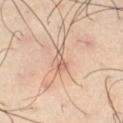follow-up = imaged on a skin check; not biopsied | body site = the right thigh | subject = male, aged 38 to 42 | lesion size = ~3 mm (longest diameter) | imaging modality = 15 mm crop, total-body photography | lighting = cross-polarized.